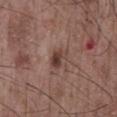Captured during whole-body skin photography for melanoma surveillance; the lesion was not biopsied. Captured under white-light illumination. The lesion's longest dimension is about 3 mm. A male subject aged approximately 60. Cropped from a whole-body photographic skin survey; the tile spans about 15 mm. Located on the chest.